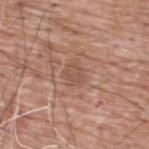Clinical impression: Captured during whole-body skin photography for melanoma surveillance; the lesion was not biopsied. Clinical summary: From the upper back. A male subject aged 58 to 62. A 15 mm crop from a total-body photograph taken for skin-cancer surveillance.